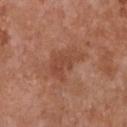Captured during whole-body skin photography for melanoma surveillance; the lesion was not biopsied. A 15 mm close-up tile from a total-body photography series done for melanoma screening. The total-body-photography lesion software estimated a lesion area of about 9.5 mm², an eccentricity of roughly 0.8, and a shape-asymmetry score of about 0.45 (0 = symmetric). And it measured an automated nevus-likeness rating near 0 out of 100. A female subject in their mid-70s. The lesion is located on the chest. Measured at roughly 4.5 mm in maximum diameter. Captured under white-light illumination.